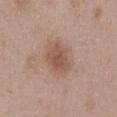The patient is a male aged 48 to 52. Cropped from a whole-body photographic skin survey; the tile spans about 15 mm. The lesion is on the abdomen. Automated tile analysis of the lesion measured a mean CIELAB color near L≈53 a*≈20 b*≈27, roughly 9 lightness units darker than nearby skin, and a normalized border contrast of about 7. And it measured an automated nevus-likeness rating near 70 out of 100 and a detector confidence of about 100 out of 100 that the crop contains a lesion. The recorded lesion diameter is about 4 mm.